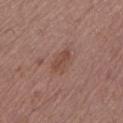A male patient about 65 years old.
A 15 mm close-up extracted from a 3D total-body photography capture.
From the leg.